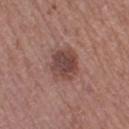Assessment:
Part of a total-body skin-imaging series; this lesion was reviewed on a skin check and was not flagged for biopsy.
Clinical summary:
A female subject, about 50 years old. From the right thigh. About 4 mm across. A 15 mm crop from a total-body photograph taken for skin-cancer surveillance. The lesion-visualizer software estimated border irregularity of about 2 on a 0–10 scale, internal color variation of about 4 on a 0–10 scale, and peripheral color asymmetry of about 1. Captured under white-light illumination.